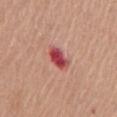{"biopsy_status": "not biopsied; imaged during a skin examination", "image": {"source": "total-body photography crop", "field_of_view_mm": 15}, "lighting": "white-light", "site": "chest", "patient": {"sex": "female", "age_approx": 65}, "automated_metrics": {"eccentricity": 0.75, "shape_asymmetry": 0.2, "cielab_L": 49, "cielab_a": 36, "cielab_b": 25, "vs_skin_darker_L": 15.0, "color_variation_0_10": 5.0, "peripheral_color_asymmetry": 1.5, "nevus_likeness_0_100": 0, "lesion_detection_confidence_0_100": 100}}